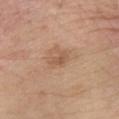Impression: Imaged during a routine full-body skin examination; the lesion was not biopsied and no histopathology is available. Acquisition and patient details: A 15 mm close-up tile from a total-body photography series done for melanoma screening. The lesion is located on the left forearm. A male patient, about 60 years old.